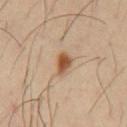Case summary:
* follow-up — total-body-photography surveillance lesion; no biopsy
* TBP lesion metrics — a footprint of about 4 mm² and two-axis asymmetry of about 0.35; a border-irregularity rating of about 3/10 and radial color variation of about 1.5; lesion-presence confidence of about 100/100
* acquisition — ~15 mm crop, total-body skin-cancer survey
* illumination — cross-polarized
* subject — male, aged 38–42
* location — the chest
* diameter — ≈3 mm